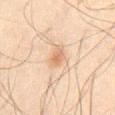Findings:
• biopsy status — catalogued during a skin exam; not biopsied
• image source — ~15 mm crop, total-body skin-cancer survey
• illumination — cross-polarized
• location — the abdomen
• diameter — about 3.5 mm
• patient — male, aged 43–47